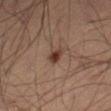Case summary:
* workup — imaged on a skin check; not biopsied
* patient — male, roughly 60 years of age
* imaging modality — ~15 mm crop, total-body skin-cancer survey
* site — the right thigh